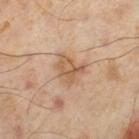The lesion was photographed on a routine skin check and not biopsied; there is no pathology result. A lesion tile, about 15 mm wide, cut from a 3D total-body photograph. Imaged with cross-polarized lighting. A male patient, about 55 years old. The lesion is on the left thigh.